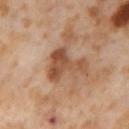Assessment:
Imaged during a routine full-body skin examination; the lesion was not biopsied and no histopathology is available.
Background:
Cropped from a whole-body photographic skin survey; the tile spans about 15 mm. A female patient, about 55 years old. Located on the leg.